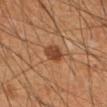Findings:
– notes: imaged on a skin check; not biopsied
– image: ~15 mm tile from a whole-body skin photo
– patient: male, aged 58 to 62
– location: the mid back